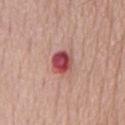notes: imaged on a skin check; not biopsied | automated lesion analysis: about 16 CIELAB-L* units darker than the surrounding skin and a normalized lesion–skin contrast near 11; a border-irregularity rating of about 1.5/10 and a within-lesion color-variation index near 7.5/10; a lesion-detection confidence of about 100/100 | image source: ~15 mm tile from a whole-body skin photo | lesion size: ~3 mm (longest diameter) | subject: male, in their mid-50s | illumination: white-light.